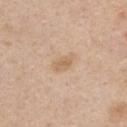| field | value |
|---|---|
| biopsy status | catalogued during a skin exam; not biopsied |
| lighting | white-light |
| site | the chest |
| image-analysis metrics | an average lesion color of about L≈64 a*≈17 b*≈34 (CIELAB), about 7 CIELAB-L* units darker than the surrounding skin, and a normalized border contrast of about 6; a border-irregularity rating of about 2/10, a within-lesion color-variation index near 1.5/10, and peripheral color asymmetry of about 0.5; an automated nevus-likeness rating near 5 out of 100 and lesion-presence confidence of about 100/100 |
| image source | ~15 mm crop, total-body skin-cancer survey |
| subject | female, roughly 45 years of age |